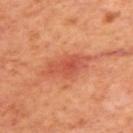Captured during whole-body skin photography for melanoma surveillance; the lesion was not biopsied. From the upper back. This image is a 15 mm lesion crop taken from a total-body photograph. This is a cross-polarized tile. A male patient, aged 68–72. Longest diameter approximately 6.5 mm.